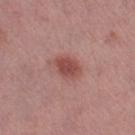No biopsy was performed on this lesion — it was imaged during a full skin examination and was not determined to be concerning. A close-up tile cropped from a whole-body skin photograph, about 15 mm across. The lesion is located on the left thigh. The subject is a female roughly 65 years of age.